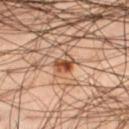Recorded during total-body skin imaging; not selected for excision or biopsy.
Cropped from a whole-body photographic skin survey; the tile spans about 15 mm.
The tile uses cross-polarized illumination.
The lesion is located on the leg.
The subject is a male in their mid- to late 40s.
The lesion's longest dimension is about 2.5 mm.
Automated tile analysis of the lesion measured an area of roughly 4.5 mm² and a symmetry-axis asymmetry near 0.35.